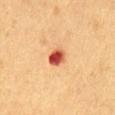notes — total-body-photography surveillance lesion; no biopsy
image-analysis metrics — an area of roughly 5 mm², an eccentricity of roughly 0.45, and a symmetry-axis asymmetry near 0.1; a lesion color around L≈50 a*≈31 b*≈35 in CIELAB, a lesion–skin lightness drop of about 17, and a normalized lesion–skin contrast near 11.5; a border-irregularity index near 1/10 and a within-lesion color-variation index near 8.5/10; a classifier nevus-likeness of about 0/100
anatomic site — the chest
patient — male, in their mid-50s
diameter — about 2.5 mm
lighting — cross-polarized illumination
image — ~15 mm crop, total-body skin-cancer survey